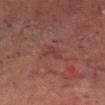Q: Was a biopsy performed?
A: imaged on a skin check; not biopsied
Q: What did automated image analysis measure?
A: an area of roughly 3 mm² and a symmetry-axis asymmetry near 0.55; an average lesion color of about L≈32 a*≈22 b*≈19 (CIELAB) and a lesion-to-skin contrast of about 5 (normalized; higher = more distinct); a border-irregularity index near 5.5/10, a within-lesion color-variation index near 0/10, and a peripheral color-asymmetry measure near 0; a classifier nevus-likeness of about 0/100 and lesion-presence confidence of about 90/100
Q: Lesion size?
A: ~3 mm (longest diameter)
Q: How was this image acquired?
A: ~15 mm crop, total-body skin-cancer survey
Q: What lighting was used for the tile?
A: cross-polarized illumination
Q: What are the patient's age and sex?
A: male, aged approximately 75
Q: Lesion location?
A: the head or neck